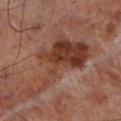{
  "biopsy_status": "not biopsied; imaged during a skin examination"
}